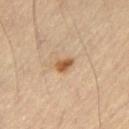The lesion was tiled from a total-body skin photograph and was not biopsied. The lesion's longest dimension is about 2.5 mm. Cropped from a whole-body photographic skin survey; the tile spans about 15 mm. The lesion is on the left thigh. Captured under cross-polarized illumination. A male patient, in their mid- to late 50s.